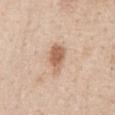Cropped from a whole-body photographic skin survey; the tile spans about 15 mm. The patient is a male in their 60s. On the chest. Approximately 3.5 mm at its widest.